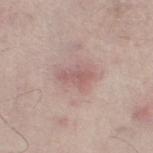Q: Is there a histopathology result?
A: catalogued during a skin exam; not biopsied
Q: What kind of image is this?
A: total-body-photography crop, ~15 mm field of view
Q: What is the anatomic site?
A: the left thigh
Q: Patient demographics?
A: male, aged around 65